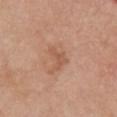Image and clinical context: A close-up tile cropped from a whole-body skin photograph, about 15 mm across. Automated image analysis of the tile measured a classifier nevus-likeness of about 0/100 and lesion-presence confidence of about 100/100. Approximately 3 mm at its widest. This is a white-light tile. A female patient aged 63–67. The lesion is on the front of the torso.